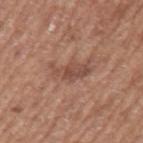No biopsy was performed on this lesion — it was imaged during a full skin examination and was not determined to be concerning.
Captured under white-light illumination.
The patient is a male in their mid- to late 70s.
This image is a 15 mm lesion crop taken from a total-body photograph.
The lesion is on the arm.